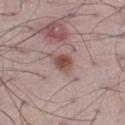notes: no biopsy performed (imaged during a skin exam) | diameter: about 2.5 mm | patient: male, aged 68–72 | lighting: white-light | imaging modality: ~15 mm crop, total-body skin-cancer survey | TBP lesion metrics: a footprint of about 4.5 mm², a shape eccentricity near 0.45, and a shape-asymmetry score of about 0.35 (0 = symmetric); a lesion color around L≈50 a*≈19 b*≈23 in CIELAB, a lesion–skin lightness drop of about 11, and a normalized border contrast of about 8.5 | site: the left thigh.